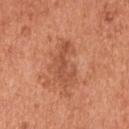Q: Was this lesion biopsied?
A: catalogued during a skin exam; not biopsied
Q: How was this image acquired?
A: total-body-photography crop, ~15 mm field of view
Q: What is the lesion's diameter?
A: about 4.5 mm
Q: Lesion location?
A: the head or neck
Q: What are the patient's age and sex?
A: male, in their mid- to late 50s